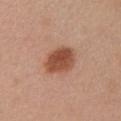Case summary:
– notes — total-body-photography surveillance lesion; no biopsy
– location — the chest
– subject — female, aged 53–57
– acquisition — ~15 mm tile from a whole-body skin photo
– image-analysis metrics — a lesion area of about 12 mm², an eccentricity of roughly 0.6, and two-axis asymmetry of about 0.15; an automated nevus-likeness rating near 100 out of 100 and a detector confidence of about 100 out of 100 that the crop contains a lesion